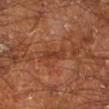Q: How was this image acquired?
A: ~15 mm tile from a whole-body skin photo
Q: What are the patient's age and sex?
A: male, roughly 65 years of age
Q: How was the tile lit?
A: cross-polarized illumination
Q: Lesion size?
A: about 3.5 mm
Q: What is the anatomic site?
A: the right lower leg